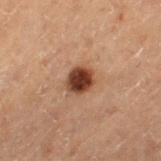<tbp_lesion>
  <biopsy_status>not biopsied; imaged during a skin examination</biopsy_status>
  <lighting>cross-polarized</lighting>
  <image>
    <source>total-body photography crop</source>
    <field_of_view_mm>15</field_of_view_mm>
  </image>
  <patient>
    <sex>male</sex>
    <age_approx>70</age_approx>
  </patient>
  <automated_metrics>
    <cielab_L>30</cielab_L>
    <cielab_a>18</cielab_a>
    <cielab_b>24</cielab_b>
    <vs_skin_darker_L>15.0</vs_skin_darker_L>
    <vs_skin_contrast_norm>13.0</vs_skin_contrast_norm>
    <border_irregularity_0_10>1.5</border_irregularity_0_10>
    <peripheral_color_asymmetry>1.0</peripheral_color_asymmetry>
  </automated_metrics>
  <site>left thigh</site>
</tbp_lesion>